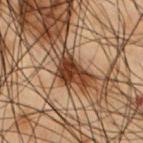Case summary:
• follow-up · no biopsy performed (imaged during a skin exam)
• image · ~15 mm tile from a whole-body skin photo
• patient · male, roughly 55 years of age
• body site · the front of the torso